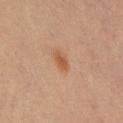follow-up: no biopsy performed (imaged during a skin exam)
patient: male, roughly 70 years of age
acquisition: ~15 mm crop, total-body skin-cancer survey
site: the abdomen